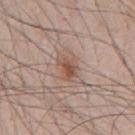biopsy_status: not biopsied; imaged during a skin examination
patient:
  sex: male
  age_approx: 45
image:
  source: total-body photography crop
  field_of_view_mm: 15
lesion_size:
  long_diameter_mm_approx: 3.5
automated_metrics:
  area_mm2_approx: 5.5
  shape_asymmetry: 0.3
  cielab_L: 53
  cielab_a: 18
  cielab_b: 26
  vs_skin_darker_L: 9.0
  vs_skin_contrast_norm: 7.0
  nevus_likeness_0_100: 90
  lesion_detection_confidence_0_100: 100
site: chest